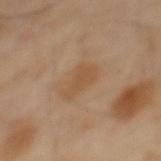Case summary:
* workup — imaged on a skin check; not biopsied
* site — the mid back
* patient — male, aged 38–42
* image — 15 mm crop, total-body photography
* illumination — cross-polarized
* automated metrics — a classifier nevus-likeness of about 15/100 and lesion-presence confidence of about 100/100